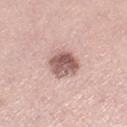Recorded during total-body skin imaging; not selected for excision or biopsy. An algorithmic analysis of the crop reported an area of roughly 9.5 mm², an eccentricity of roughly 0.4, and a shape-asymmetry score of about 0.2 (0 = symmetric). The software also gave a border-irregularity rating of about 2/10, a within-lesion color-variation index near 6/10, and radial color variation of about 2. A female patient, in their mid- to late 60s. Measured at roughly 4 mm in maximum diameter. Cropped from a total-body skin-imaging series; the visible field is about 15 mm. Located on the right thigh. Captured under white-light illumination.